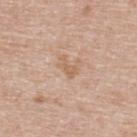Q: Is there a histopathology result?
A: catalogued during a skin exam; not biopsied
Q: What is the anatomic site?
A: the upper back
Q: Patient demographics?
A: female, aged 38–42
Q: Illumination type?
A: white-light
Q: What is the imaging modality?
A: ~15 mm crop, total-body skin-cancer survey
Q: What did automated image analysis measure?
A: a lesion area of about 2.5 mm² and an outline eccentricity of about 0.85 (0 = round, 1 = elongated); a within-lesion color-variation index near 0/10 and radial color variation of about 0; a nevus-likeness score of about 0/100